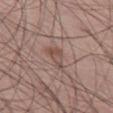Impression: Part of a total-body skin-imaging series; this lesion was reviewed on a skin check and was not flagged for biopsy. Clinical summary: Cropped from a total-body skin-imaging series; the visible field is about 15 mm. Imaged with white-light lighting. From the leg. A male patient aged 58 to 62. Measured at roughly 3.5 mm in maximum diameter. The total-body-photography lesion software estimated a lesion area of about 4 mm² and a shape-asymmetry score of about 0.4 (0 = symmetric). It also reported an automated nevus-likeness rating near 5 out of 100 and a detector confidence of about 100 out of 100 that the crop contains a lesion.